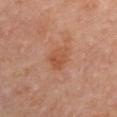Acquisition and patient details: Automated image analysis of the tile measured a footprint of about 6.5 mm², an outline eccentricity of about 0.7 (0 = round, 1 = elongated), and two-axis asymmetry of about 0.25. It also reported an average lesion color of about L≈50 a*≈24 b*≈33 (CIELAB), about 7 CIELAB-L* units darker than the surrounding skin, and a lesion-to-skin contrast of about 6 (normalized; higher = more distinct). And it measured a border-irregularity rating of about 3/10 and radial color variation of about 1.5. The analysis additionally found a nevus-likeness score of about 0/100 and lesion-presence confidence of about 100/100. On the left upper arm. A female patient approximately 50 years of age. Cropped from a total-body skin-imaging series; the visible field is about 15 mm.